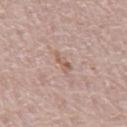Impression:
No biopsy was performed on this lesion — it was imaged during a full skin examination and was not determined to be concerning.
Context:
This image is a 15 mm lesion crop taken from a total-body photograph. Captured under white-light illumination. A male subject aged around 70. Approximately 3 mm at its widest. The lesion is on the front of the torso.